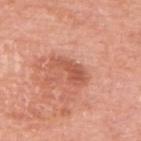Q: Was a biopsy performed?
A: catalogued during a skin exam; not biopsied
Q: How was the tile lit?
A: white-light
Q: What is the anatomic site?
A: the upper back
Q: How was this image acquired?
A: ~15 mm tile from a whole-body skin photo
Q: Who is the patient?
A: female, in their mid-70s
Q: How large is the lesion?
A: about 4.5 mm